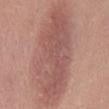Acquisition and patient details: The patient is a male aged approximately 30. About 14 mm across. Located on the front of the torso. A close-up tile cropped from a whole-body skin photograph, about 15 mm across. The total-body-photography lesion software estimated an area of roughly 55 mm², a shape eccentricity near 0.95, and two-axis asymmetry of about 0.15. The software also gave a mean CIELAB color near L≈54 a*≈23 b*≈24, a lesion–skin lightness drop of about 9, and a normalized lesion–skin contrast near 6.5. And it measured a nevus-likeness score of about 5/100 and lesion-presence confidence of about 100/100. Captured under white-light illumination.